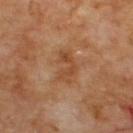The lesion was tiled from a total-body skin photograph and was not biopsied. A 15 mm crop from a total-body photograph taken for skin-cancer surveillance. This is a cross-polarized tile. The lesion is on the upper back. The subject is a male aged 68 to 72. The lesion's longest dimension is about 3.5 mm.